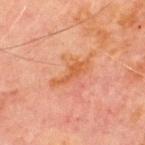| feature | finding |
|---|---|
| notes | no biopsy performed (imaged during a skin exam) |
| patient | male, aged 68 to 72 |
| lighting | cross-polarized |
| image source | 15 mm crop, total-body photography |
| TBP lesion metrics | an average lesion color of about L≈48 a*≈25 b*≈35 (CIELAB) and a lesion-to-skin contrast of about 7 (normalized; higher = more distinct) |
| lesion diameter | ~5 mm (longest diameter) |
| site | the chest |